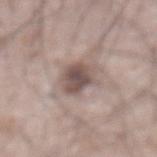workup: imaged on a skin check; not biopsied | tile lighting: white-light illumination | automated lesion analysis: a footprint of about 9 mm², an outline eccentricity of about 0.6 (0 = round, 1 = elongated), and a shape-asymmetry score of about 0.2 (0 = symmetric); an average lesion color of about L≈51 a*≈15 b*≈19 (CIELAB), roughly 13 lightness units darker than nearby skin, and a normalized lesion–skin contrast near 9.5; a border-irregularity rating of about 2.5/10, a color-variation rating of about 5/10, and radial color variation of about 1.5; a classifier nevus-likeness of about 60/100 and a lesion-detection confidence of about 85/100 | site: the abdomen | image source: ~15 mm crop, total-body skin-cancer survey | patient: male, aged approximately 65.